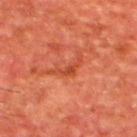Notes:
* follow-up: catalogued during a skin exam; not biopsied
* patient: male, aged around 65
* body site: the back
* image: total-body-photography crop, ~15 mm field of view